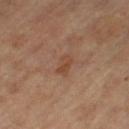Recorded during total-body skin imaging; not selected for excision or biopsy.
From the left thigh.
The patient is a female approximately 65 years of age.
The tile uses cross-polarized illumination.
The total-body-photography lesion software estimated a lesion area of about 3.5 mm², an eccentricity of roughly 0.85, and two-axis asymmetry of about 0.25. The software also gave a lesion color around L≈44 a*≈20 b*≈30 in CIELAB, roughly 7 lightness units darker than nearby skin, and a normalized border contrast of about 6. It also reported a classifier nevus-likeness of about 0/100 and a lesion-detection confidence of about 100/100.
Measured at roughly 3 mm in maximum diameter.
This image is a 15 mm lesion crop taken from a total-body photograph.